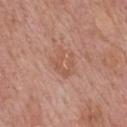Captured during whole-body skin photography for melanoma surveillance; the lesion was not biopsied.
Automated image analysis of the tile measured a mean CIELAB color near L≈56 a*≈23 b*≈29. The analysis additionally found an automated nevus-likeness rating near 0 out of 100.
Imaged with white-light lighting.
A male patient roughly 70 years of age.
The lesion is on the chest.
A 15 mm close-up extracted from a 3D total-body photography capture.